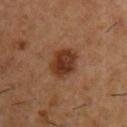| field | value |
|---|---|
| image | ~15 mm crop, total-body skin-cancer survey |
| automated metrics | an area of roughly 9 mm², an eccentricity of roughly 0.6, and a shape-asymmetry score of about 0.15 (0 = symmetric); a nevus-likeness score of about 95/100 and a detector confidence of about 100 out of 100 that the crop contains a lesion |
| diameter | about 4 mm |
| lighting | cross-polarized |
| site | the chest |
| subject | male, approximately 55 years of age |